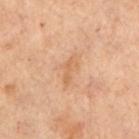Clinical impression: Recorded during total-body skin imaging; not selected for excision or biopsy. Context: The lesion is located on the chest. A female patient about 55 years old. Cropped from a whole-body photographic skin survey; the tile spans about 15 mm.